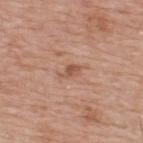- follow-up: total-body-photography surveillance lesion; no biopsy
- site: the upper back
- size: ~2.5 mm (longest diameter)
- tile lighting: white-light illumination
- automated metrics: an area of roughly 2.5 mm², an outline eccentricity of about 0.8 (0 = round, 1 = elongated), and a shape-asymmetry score of about 0.35 (0 = symmetric); a lesion color around L≈54 a*≈22 b*≈30 in CIELAB, about 9 CIELAB-L* units darker than the surrounding skin, and a normalized border contrast of about 6.5
- patient: male, in their mid-70s
- acquisition: ~15 mm tile from a whole-body skin photo